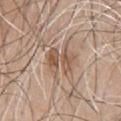image:
  source: total-body photography crop
  field_of_view_mm: 15
lesion_size:
  long_diameter_mm_approx: 3.5
site: chest
automated_metrics:
  eccentricity: 0.7
  nevus_likeness_0_100: 0
  lesion_detection_confidence_0_100: 80
lighting: white-light
patient:
  sex: male
  age_approx: 65